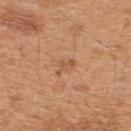Q: Was a biopsy performed?
A: total-body-photography surveillance lesion; no biopsy
Q: Illumination type?
A: white-light
Q: Patient demographics?
A: male, aged 43–47
Q: Lesion location?
A: the back
Q: How was this image acquired?
A: ~15 mm tile from a whole-body skin photo
Q: What did automated image analysis measure?
A: an outline eccentricity of about 0.85 (0 = round, 1 = elongated) and a symmetry-axis asymmetry near 0.5; an average lesion color of about L≈55 a*≈23 b*≈35 (CIELAB), roughly 8 lightness units darker than nearby skin, and a normalized lesion–skin contrast near 5.5; border irregularity of about 5.5 on a 0–10 scale, a within-lesion color-variation index near 0/10, and a peripheral color-asymmetry measure near 0